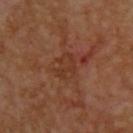Clinical impression:
Imaged during a routine full-body skin examination; the lesion was not biopsied and no histopathology is available.
Image and clinical context:
A 15 mm close-up extracted from a 3D total-body photography capture. A male patient, aged around 55. An algorithmic analysis of the crop reported an area of roughly 6 mm², an outline eccentricity of about 0.6 (0 = round, 1 = elongated), and a symmetry-axis asymmetry near 0.25. The analysis additionally found a mean CIELAB color near L≈34 a*≈22 b*≈30 and about 5 CIELAB-L* units darker than the surrounding skin. About 3 mm across. This is a cross-polarized tile. The lesion is on the upper back.